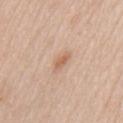Q: Was this lesion biopsied?
A: no biopsy performed (imaged during a skin exam)
Q: What is the lesion's diameter?
A: about 2.5 mm
Q: Who is the patient?
A: male, approximately 60 years of age
Q: How was this image acquired?
A: total-body-photography crop, ~15 mm field of view
Q: Where on the body is the lesion?
A: the chest
Q: Automated lesion metrics?
A: a lesion color around L≈62 a*≈20 b*≈32 in CIELAB; a classifier nevus-likeness of about 25/100
Q: What lighting was used for the tile?
A: white-light